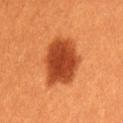{
  "biopsy_status": "not biopsied; imaged during a skin examination",
  "site": "lower back",
  "patient": {
    "sex": "female",
    "age_approx": 30
  },
  "image": {
    "source": "total-body photography crop",
    "field_of_view_mm": 15
  }
}